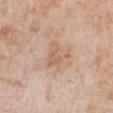biopsy status = catalogued during a skin exam; not biopsied
lighting = white-light illumination
automated lesion analysis = an eccentricity of roughly 0.4 and two-axis asymmetry of about 0.5; an average lesion color of about L≈62 a*≈18 b*≈31 (CIELAB) and a lesion–skin lightness drop of about 7; a border-irregularity index near 5.5/10 and a within-lesion color-variation index near 2/10; a lesion-detection confidence of about 100/100
imaging modality = total-body-photography crop, ~15 mm field of view
location = the right lower leg
lesion diameter = about 3 mm
patient = female, about 70 years old